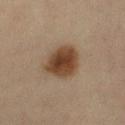Captured during whole-body skin photography for melanoma surveillance; the lesion was not biopsied. Automated image analysis of the tile measured a within-lesion color-variation index near 3.5/10. The analysis additionally found a classifier nevus-likeness of about 100/100 and a lesion-detection confidence of about 100/100. The recorded lesion diameter is about 4.5 mm. The subject is a female approximately 45 years of age. Imaged with cross-polarized lighting. A close-up tile cropped from a whole-body skin photograph, about 15 mm across. The lesion is located on the right thigh.